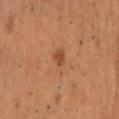| key | value |
|---|---|
| workup | catalogued during a skin exam; not biopsied |
| site | the head or neck |
| lesion diameter | ~3 mm (longest diameter) |
| acquisition | 15 mm crop, total-body photography |
| subject | male, aged around 55 |